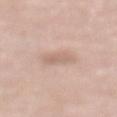Automated tile analysis of the lesion measured a lesion color around L≈65 a*≈17 b*≈26 in CIELAB, a lesion–skin lightness drop of about 7, and a normalized lesion–skin contrast near 5. And it measured internal color variation of about 2 on a 0–10 scale and radial color variation of about 0.5. And it measured a classifier nevus-likeness of about 10/100 and lesion-presence confidence of about 100/100.
The lesion is on the chest.
A female patient, aged approximately 65.
The recorded lesion diameter is about 3.5 mm.
The tile uses white-light illumination.
A 15 mm close-up tile from a total-body photography series done for melanoma screening.